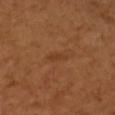Q: Is there a histopathology result?
A: imaged on a skin check; not biopsied
Q: How large is the lesion?
A: ≈2.5 mm
Q: Lesion location?
A: the right forearm
Q: How was this image acquired?
A: total-body-photography crop, ~15 mm field of view
Q: What are the patient's age and sex?
A: female, in their mid-50s
Q: Illumination type?
A: cross-polarized